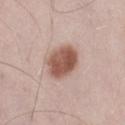lesion diameter — ~4.5 mm (longest diameter) | automated metrics — a footprint of about 12 mm²; an average lesion color of about L≈54 a*≈21 b*≈26 (CIELAB), a lesion–skin lightness drop of about 16, and a lesion-to-skin contrast of about 10.5 (normalized; higher = more distinct); a color-variation rating of about 4.5/10 and radial color variation of about 1.5 | image source — 15 mm crop, total-body photography | tile lighting — white-light | patient — male, roughly 55 years of age | body site — the leg.